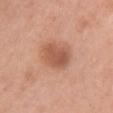Clinical impression:
No biopsy was performed on this lesion — it was imaged during a full skin examination and was not determined to be concerning.
Acquisition and patient details:
Automated tile analysis of the lesion measured a lesion color around L≈55 a*≈24 b*≈32 in CIELAB, roughly 11 lightness units darker than nearby skin, and a lesion-to-skin contrast of about 7 (normalized; higher = more distinct). The software also gave a border-irregularity index near 1.5/10, internal color variation of about 3 on a 0–10 scale, and peripheral color asymmetry of about 1. A region of skin cropped from a whole-body photographic capture, roughly 15 mm wide. The patient is a female aged 33–37. The lesion is on the front of the torso.